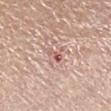Impression:
Recorded during total-body skin imaging; not selected for excision or biopsy.
Background:
A male patient, in their mid- to late 50s. Cropped from a total-body skin-imaging series; the visible field is about 15 mm. Measured at roughly 2.5 mm in maximum diameter. The lesion is located on the right lower leg.